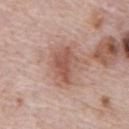Notes:
• follow-up — catalogued during a skin exam; not biopsied
• patient — male, roughly 70 years of age
• lighting — white-light illumination
• location — the abdomen
• acquisition — total-body-photography crop, ~15 mm field of view
• diameter — about 4.5 mm